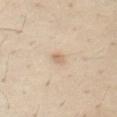Findings:
- notes: no biopsy performed (imaged during a skin exam)
- imaging modality: 15 mm crop, total-body photography
- lesion diameter: about 1.5 mm
- patient: male, approximately 30 years of age
- tile lighting: cross-polarized
- anatomic site: the front of the torso